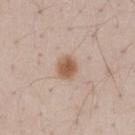Case summary:
• workup — no biopsy performed (imaged during a skin exam)
• image — ~15 mm crop, total-body skin-cancer survey
• anatomic site — the chest
• subject — male, about 50 years old